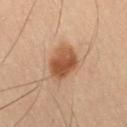Q: Was a biopsy performed?
A: catalogued during a skin exam; not biopsied
Q: What are the patient's age and sex?
A: male, approximately 55 years of age
Q: Illumination type?
A: cross-polarized
Q: What is the lesion's diameter?
A: about 3.5 mm
Q: Automated lesion metrics?
A: a shape eccentricity near 0.35 and a symmetry-axis asymmetry near 0.25; a mean CIELAB color near L≈41 a*≈19 b*≈29, roughly 11 lightness units darker than nearby skin, and a normalized border contrast of about 9.5; a color-variation rating of about 4/10 and peripheral color asymmetry of about 1.5; a classifier nevus-likeness of about 100/100 and a detector confidence of about 100 out of 100 that the crop contains a lesion
Q: Lesion location?
A: the leg
Q: How was this image acquired?
A: 15 mm crop, total-body photography